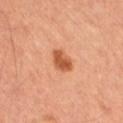follow-up = no biopsy performed (imaged during a skin exam)
patient = female
tile lighting = cross-polarized
location = the right thigh
size = ≈3 mm
TBP lesion metrics = an area of roughly 5.5 mm², a shape eccentricity near 0.75, and a symmetry-axis asymmetry near 0.25; a border-irregularity rating of about 2.5/10 and internal color variation of about 2.5 on a 0–10 scale; a lesion-detection confidence of about 100/100
image source = 15 mm crop, total-body photography